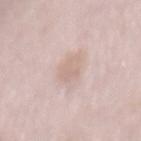Notes:
– biopsy status: total-body-photography surveillance lesion; no biopsy
– patient: female, aged around 50
– image: ~15 mm crop, total-body skin-cancer survey
– lesion size: about 4.5 mm
– site: the mid back
– TBP lesion metrics: an eccentricity of roughly 0.9 and two-axis asymmetry of about 0.3; a border-irregularity rating of about 3.5/10, a color-variation rating of about 2.5/10, and peripheral color asymmetry of about 1